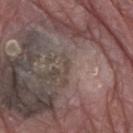site: the right upper arm | TBP lesion metrics: a footprint of about 1 mm² and a symmetry-axis asymmetry near 0.55; a border-irregularity index near 5/10; an automated nevus-likeness rating near 0 out of 100 and a lesion-detection confidence of about 0/100 | size: ~1.5 mm (longest diameter) | lighting: white-light | subject: male, aged 78 to 82 | imaging modality: ~15 mm crop, total-body skin-cancer survey.